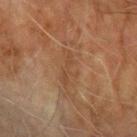workup = catalogued during a skin exam; not biopsied | image = ~15 mm crop, total-body skin-cancer survey | lesion diameter = ≈4 mm | lighting = cross-polarized illumination | subject = male, roughly 70 years of age | site = the left forearm.